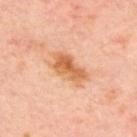follow-up: total-body-photography surveillance lesion; no biopsy
anatomic site: the upper back
lesion diameter: ≈5 mm
imaging modality: ~15 mm tile from a whole-body skin photo
automated metrics: an area of roughly 11 mm², an eccentricity of roughly 0.85, and a shape-asymmetry score of about 0.25 (0 = symmetric); border irregularity of about 3 on a 0–10 scale, a within-lesion color-variation index near 5/10, and a peripheral color-asymmetry measure near 1.5; an automated nevus-likeness rating near 70 out of 100 and lesion-presence confidence of about 100/100
patient: aged 53 to 57